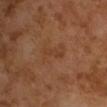biopsy status = total-body-photography surveillance lesion; no biopsy | lesion diameter = about 3.5 mm | patient = male, aged 63 to 67 | TBP lesion metrics = an area of roughly 2.5 mm² and a shape eccentricity near 0.95; a within-lesion color-variation index near 0/10 | image source = ~15 mm crop, total-body skin-cancer survey | tile lighting = cross-polarized illumination.